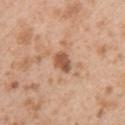The lesion was tiled from a total-body skin photograph and was not biopsied. Imaged with white-light lighting. Approximately 2.5 mm at its widest. Cropped from a whole-body photographic skin survey; the tile spans about 15 mm. The lesion is located on the upper back. Automated tile analysis of the lesion measured an eccentricity of roughly 0.65 and a shape-asymmetry score of about 0.25 (0 = symmetric). And it measured an average lesion color of about L≈54 a*≈23 b*≈33 (CIELAB), a lesion–skin lightness drop of about 13, and a normalized lesion–skin contrast near 8.5. The analysis additionally found border irregularity of about 2 on a 0–10 scale and a peripheral color-asymmetry measure near 1. And it measured an automated nevus-likeness rating near 45 out of 100 and a detector confidence of about 100 out of 100 that the crop contains a lesion. The subject is a male roughly 25 years of age.